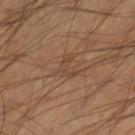{"biopsy_status": "not biopsied; imaged during a skin examination", "patient": {"sex": "male", "age_approx": 40}, "image": {"source": "total-body photography crop", "field_of_view_mm": 15}, "site": "right lower leg"}